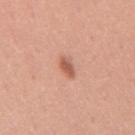follow-up: no biopsy performed (imaged during a skin exam)
subject: male, about 30 years old
diameter: ≈2.5 mm
image: 15 mm crop, total-body photography
lighting: white-light
site: the back
image-analysis metrics: a border-irregularity index near 2.5/10